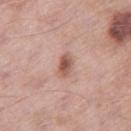biopsy status = imaged on a skin check; not biopsied